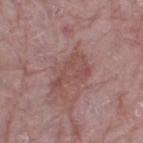Case summary:
- notes · imaged on a skin check; not biopsied
- image source · total-body-photography crop, ~15 mm field of view
- location · the leg
- patient · female, aged around 65
- diameter · ≈5 mm
- TBP lesion metrics · an area of roughly 8.5 mm², a shape eccentricity near 0.85, and a shape-asymmetry score of about 0.55 (0 = symmetric); a lesion color around L≈49 a*≈22 b*≈22 in CIELAB and roughly 7 lightness units darker than nearby skin; border irregularity of about 6 on a 0–10 scale, internal color variation of about 3.5 on a 0–10 scale, and radial color variation of about 1
- tile lighting · white-light